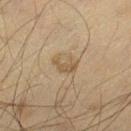The lesion was tiled from a total-body skin photograph and was not biopsied. The lesion is on the left thigh. This is a cross-polarized tile. A close-up tile cropped from a whole-body skin photograph, about 15 mm across. A male patient about 50 years old. The recorded lesion diameter is about 3 mm.